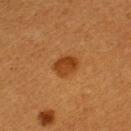| field | value |
|---|---|
| follow-up | catalogued during a skin exam; not biopsied |
| subject | female, about 55 years old |
| imaging modality | 15 mm crop, total-body photography |
| site | the left upper arm |